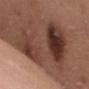Clinical impression:
Imaged during a routine full-body skin examination; the lesion was not biopsied and no histopathology is available.
Image and clinical context:
The lesion is on the front of the torso. Cropped from a total-body skin-imaging series; the visible field is about 15 mm. The patient is a female aged around 50. The tile uses white-light illumination. About 11 mm across.